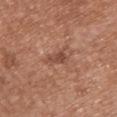Clinical impression: This lesion was catalogued during total-body skin photography and was not selected for biopsy. Acquisition and patient details: A close-up tile cropped from a whole-body skin photograph, about 15 mm across. This is a white-light tile. A female subject, aged 38–42. The lesion is located on the upper back.